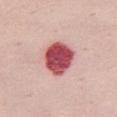Impression:
No biopsy was performed on this lesion — it was imaged during a full skin examination and was not determined to be concerning.
Context:
Captured under white-light illumination. A 15 mm close-up extracted from a 3D total-body photography capture. The patient is a female roughly 50 years of age. The recorded lesion diameter is about 4.5 mm. The lesion is located on the mid back.